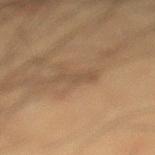Case summary:
– notes: catalogued during a skin exam; not biopsied
– location: the left lower leg
– diameter: ~3.5 mm (longest diameter)
– tile lighting: cross-polarized
– TBP lesion metrics: a mean CIELAB color near L≈44 a*≈13 b*≈28 and a lesion-to-skin contrast of about 4.5 (normalized; higher = more distinct); a classifier nevus-likeness of about 0/100 and lesion-presence confidence of about 75/100
– imaging modality: total-body-photography crop, ~15 mm field of view
– patient: male, about 35 years old